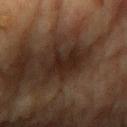Q: Was this lesion biopsied?
A: total-body-photography surveillance lesion; no biopsy
Q: Where on the body is the lesion?
A: the chest
Q: Illumination type?
A: cross-polarized
Q: What are the patient's age and sex?
A: male, aged around 85 or older
Q: Automated lesion metrics?
A: a normalized lesion–skin contrast near 9.5; an automated nevus-likeness rating near 15 out of 100
Q: What is the imaging modality?
A: ~15 mm crop, total-body skin-cancer survey
Q: Lesion size?
A: ~6 mm (longest diameter)